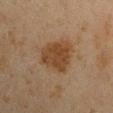Captured during whole-body skin photography for melanoma surveillance; the lesion was not biopsied.